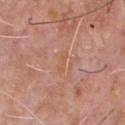subject=male, roughly 60 years of age | body site=the chest | lighting=white-light illumination | lesion diameter=about 3 mm | acquisition=~15 mm crop, total-body skin-cancer survey.